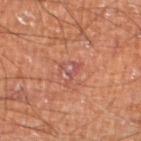Clinical impression:
No biopsy was performed on this lesion — it was imaged during a full skin examination and was not determined to be concerning.
Image and clinical context:
A roughly 15 mm field-of-view crop from a total-body skin photograph. A male subject, approximately 60 years of age. Longest diameter approximately 3 mm. Imaged with cross-polarized lighting. The lesion is on the left lower leg.